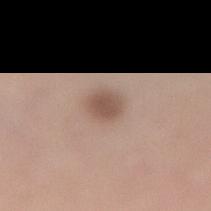follow-up — no biopsy performed (imaged during a skin exam) | TBP lesion metrics — a lesion color around L≈53 a*≈17 b*≈25 in CIELAB, roughly 11 lightness units darker than nearby skin, and a lesion-to-skin contrast of about 7.5 (normalized; higher = more distinct); a color-variation rating of about 2/10 and peripheral color asymmetry of about 0.5; a lesion-detection confidence of about 100/100 | illumination — white-light illumination | diameter — about 2.5 mm | image — ~15 mm crop, total-body skin-cancer survey | patient — male, aged 58 to 62 | location — the leg.